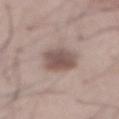biopsy_status: not biopsied; imaged during a skin examination
image:
  source: total-body photography crop
  field_of_view_mm: 15
site: abdomen
lighting: white-light
patient:
  sex: male
  age_approx: 55
lesion_size:
  long_diameter_mm_approx: 4.5